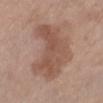workup: no biopsy performed (imaged during a skin exam)
image: total-body-photography crop, ~15 mm field of view
body site: the left lower leg
TBP lesion metrics: an average lesion color of about L≈52 a*≈19 b*≈27 (CIELAB), a lesion–skin lightness drop of about 9, and a normalized border contrast of about 6.5; a peripheral color-asymmetry measure near 1
patient: female, aged around 65
size: ≈7.5 mm
lighting: white-light